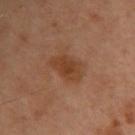The lesion was tiled from a total-body skin photograph and was not biopsied. Located on the upper back. A lesion tile, about 15 mm wide, cut from a 3D total-body photograph. A female patient about 55 years old.